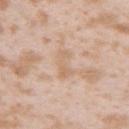Impression: No biopsy was performed on this lesion — it was imaged during a full skin examination and was not determined to be concerning. Background: A female subject roughly 25 years of age. A 15 mm close-up extracted from a 3D total-body photography capture. The lesion is on the left upper arm. The tile uses white-light illumination.